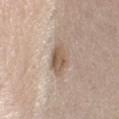workup — total-body-photography surveillance lesion; no biopsy
patient — female, aged 58 to 62
illumination — white-light illumination
imaging modality — ~15 mm crop, total-body skin-cancer survey
lesion size — about 4.5 mm
location — the left lower leg